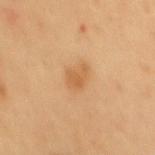Recorded during total-body skin imaging; not selected for excision or biopsy. Approximately 3 mm at its widest. A male subject, aged around 50. A close-up tile cropped from a whole-body skin photograph, about 15 mm across. Automated tile analysis of the lesion measured an area of roughly 4.5 mm², an eccentricity of roughly 0.8, and a shape-asymmetry score of about 0.35 (0 = symmetric). And it measured an average lesion color of about L≈59 a*≈22 b*≈41 (CIELAB) and a normalized border contrast of about 6. The software also gave a border-irregularity index near 3.5/10, a within-lesion color-variation index near 1.5/10, and peripheral color asymmetry of about 0.5. From the back.